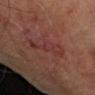Impression:
Captured during whole-body skin photography for melanoma surveillance; the lesion was not biopsied.